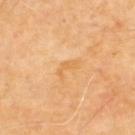Notes:
– workup · imaged on a skin check; not biopsied